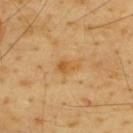Imaged during a routine full-body skin examination; the lesion was not biopsied and no histopathology is available.
On the upper back.
A lesion tile, about 15 mm wide, cut from a 3D total-body photograph.
A male patient, about 65 years old.
Automated image analysis of the tile measured a lesion area of about 3 mm², a shape eccentricity near 0.8, and a shape-asymmetry score of about 0.45 (0 = symmetric). It also reported a lesion color around L≈58 a*≈21 b*≈47 in CIELAB, about 8 CIELAB-L* units darker than the surrounding skin, and a normalized border contrast of about 7. The analysis additionally found a nevus-likeness score of about 0/100 and a detector confidence of about 100 out of 100 that the crop contains a lesion.
Captured under cross-polarized illumination.